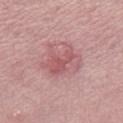follow-up: no biopsy performed (imaged during a skin exam) | patient: female, roughly 45 years of age | location: the right thigh | tile lighting: white-light illumination | image: ~15 mm tile from a whole-body skin photo.